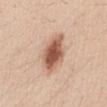Q: Was a biopsy performed?
A: imaged on a skin check; not biopsied
Q: Who is the patient?
A: female, aged approximately 25
Q: What is the anatomic site?
A: the mid back
Q: What did automated image analysis measure?
A: an average lesion color of about L≈57 a*≈22 b*≈30 (CIELAB), roughly 16 lightness units darker than nearby skin, and a normalized border contrast of about 10.5; an automated nevus-likeness rating near 100 out of 100 and a lesion-detection confidence of about 100/100
Q: How was the tile lit?
A: white-light illumination
Q: How was this image acquired?
A: ~15 mm crop, total-body skin-cancer survey
Q: How large is the lesion?
A: ≈5 mm